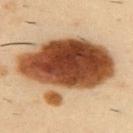Assessment:
Imaged during a routine full-body skin examination; the lesion was not biopsied and no histopathology is available.
Context:
A 15 mm close-up extracted from a 3D total-body photography capture. The lesion is on the back. A male patient aged around 40.